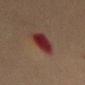The lesion was photographed on a routine skin check and not biopsied; there is no pathology result. A female patient about 70 years old. The lesion is located on the mid back. A lesion tile, about 15 mm wide, cut from a 3D total-body photograph.